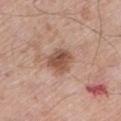{"biopsy_status": "not biopsied; imaged during a skin examination", "patient": {"sex": "male", "age_approx": 80}, "site": "left lower leg", "image": {"source": "total-body photography crop", "field_of_view_mm": 15}}